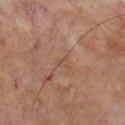Imaged during a routine full-body skin examination; the lesion was not biopsied and no histopathology is available.
The lesion is on the chest.
Imaged with cross-polarized lighting.
An algorithmic analysis of the crop reported a shape eccentricity near 0.65 and a symmetry-axis asymmetry near 0.45. The software also gave a mean CIELAB color near L≈48 a*≈20 b*≈28, a lesion–skin lightness drop of about 5, and a normalized lesion–skin contrast near 4. It also reported a border-irregularity index near 3.5/10, a color-variation rating of about 0/10, and peripheral color asymmetry of about 0.
The recorded lesion diameter is about 1.5 mm.
A male subject aged 68 to 72.
Cropped from a whole-body photographic skin survey; the tile spans about 15 mm.